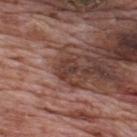Assessment: The lesion was tiled from a total-body skin photograph and was not biopsied. Context: The subject is a male aged around 70. The tile uses white-light illumination. From the upper back. Cropped from a total-body skin-imaging series; the visible field is about 15 mm.